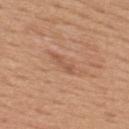Case summary:
- notes: no biopsy performed (imaged during a skin exam)
- size: about 3 mm
- lighting: white-light illumination
- image-analysis metrics: a lesion area of about 2.5 mm², a shape eccentricity near 0.95, and a symmetry-axis asymmetry near 0.35; a normalized border contrast of about 5.5; a border-irregularity rating of about 4/10 and internal color variation of about 0 on a 0–10 scale
- site: the upper back
- subject: male, approximately 50 years of age
- imaging modality: ~15 mm tile from a whole-body skin photo